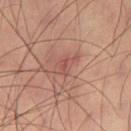Impression: No biopsy was performed on this lesion — it was imaged during a full skin examination and was not determined to be concerning. Clinical summary: A region of skin cropped from a whole-body photographic capture, roughly 15 mm wide. The tile uses cross-polarized illumination. From the leg. The patient is a male roughly 40 years of age. Automated image analysis of the tile measured a lesion color around L≈50 a*≈24 b*≈24 in CIELAB, roughly 7 lightness units darker than nearby skin, and a lesion-to-skin contrast of about 5.5 (normalized; higher = more distinct).